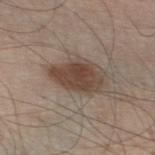{
  "site": "left thigh",
  "lighting": "white-light",
  "lesion_size": {
    "long_diameter_mm_approx": 5.5
  },
  "image": {
    "source": "total-body photography crop",
    "field_of_view_mm": 15
  },
  "patient": {
    "sex": "male",
    "age_approx": 60
  }
}